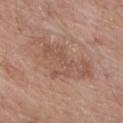A region of skin cropped from a whole-body photographic capture, roughly 15 mm wide. A male subject, roughly 65 years of age. From the front of the torso.